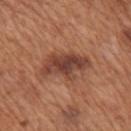Q: Was a biopsy performed?
A: no biopsy performed (imaged during a skin exam)
Q: What is the anatomic site?
A: the mid back
Q: How large is the lesion?
A: ~6 mm (longest diameter)
Q: What is the imaging modality?
A: ~15 mm crop, total-body skin-cancer survey
Q: What are the patient's age and sex?
A: male, approximately 65 years of age
Q: How was the tile lit?
A: white-light illumination